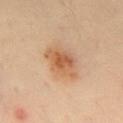<record>
  <site>back</site>
  <automated_metrics>
    <area_mm2_approx>10.0</area_mm2_approx>
    <eccentricity>0.85</eccentricity>
    <shape_asymmetry>0.25</shape_asymmetry>
    <nevus_likeness_0_100>95</nevus_likeness_0_100>
    <lesion_detection_confidence_0_100>100</lesion_detection_confidence_0_100>
  </automated_metrics>
  <image>
    <source>total-body photography crop</source>
    <field_of_view_mm>15</field_of_view_mm>
  </image>
  <lighting>cross-polarized</lighting>
  <patient>
    <sex>male</sex>
    <age_approx>50</age_approx>
  </patient>
  <lesion_size>
    <long_diameter_mm_approx>5.0</long_diameter_mm_approx>
  </lesion_size>
</record>